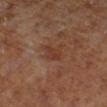anatomic site: the right lower leg
diameter: ≈3 mm
subject: female
lighting: cross-polarized illumination
imaging modality: 15 mm crop, total-body photography